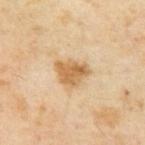No biopsy was performed on this lesion — it was imaged during a full skin examination and was not determined to be concerning. A male patient, about 65 years old. Approximately 4 mm at its widest. A 15 mm close-up extracted from a 3D total-body photography capture.